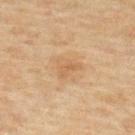biopsy status: no biopsy performed (imaged during a skin exam) | lesion diameter: ~3 mm (longest diameter) | acquisition: ~15 mm crop, total-body skin-cancer survey | lighting: cross-polarized illumination | subject: male, aged 43 to 47 | body site: the upper back | automated lesion analysis: a lesion color around L≈52 a*≈16 b*≈33 in CIELAB and a lesion-to-skin contrast of about 5 (normalized; higher = more distinct); a border-irregularity rating of about 4.5/10, internal color variation of about 1.5 on a 0–10 scale, and a peripheral color-asymmetry measure near 0.5.